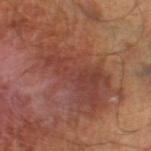* follow-up — catalogued during a skin exam; not biopsied
* location — the right lower leg
* patient — male, roughly 45 years of age
* imaging modality — ~15 mm crop, total-body skin-cancer survey